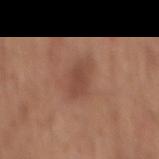Captured during whole-body skin photography for melanoma surveillance; the lesion was not biopsied. The lesion's longest dimension is about 3.5 mm. A roughly 15 mm field-of-view crop from a total-body skin photograph. From the mid back. Captured under white-light illumination. A male subject approximately 60 years of age.